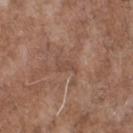<record>
  <biopsy_status>not biopsied; imaged during a skin examination</biopsy_status>
  <lesion_size>
    <long_diameter_mm_approx>2.5</long_diameter_mm_approx>
  </lesion_size>
  <patient>
    <sex>male</sex>
    <age_approx>70</age_approx>
  </patient>
  <lighting>white-light</lighting>
  <site>chest</site>
  <image>
    <source>total-body photography crop</source>
    <field_of_view_mm>15</field_of_view_mm>
  </image>
</record>